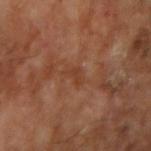{
  "biopsy_status": "not biopsied; imaged during a skin examination",
  "lighting": "cross-polarized",
  "image": {
    "source": "total-body photography crop",
    "field_of_view_mm": 15
  },
  "site": "arm",
  "patient": {
    "sex": "male",
    "age_approx": 65
  }
}